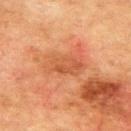The lesion was photographed on a routine skin check and not biopsied; there is no pathology result.
A roughly 15 mm field-of-view crop from a total-body skin photograph.
Imaged with cross-polarized lighting.
A male subject aged approximately 75.
Automated tile analysis of the lesion measured a footprint of about 9.5 mm² and an eccentricity of roughly 0.85. The software also gave an average lesion color of about L≈46 a*≈25 b*≈34 (CIELAB), about 7 CIELAB-L* units darker than the surrounding skin, and a normalized border contrast of about 5.5. And it measured border irregularity of about 3 on a 0–10 scale, internal color variation of about 3.5 on a 0–10 scale, and radial color variation of about 1.
Measured at roughly 5 mm in maximum diameter.
From the upper back.